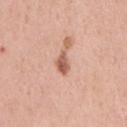{"biopsy_status": "not biopsied; imaged during a skin examination", "patient": {"sex": "male", "age_approx": 55}, "image": {"source": "total-body photography crop", "field_of_view_mm": 15}, "site": "front of the torso", "automated_metrics": {"area_mm2_approx": 3.5}, "lesion_size": {"long_diameter_mm_approx": 3.0}}